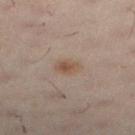Captured during whole-body skin photography for melanoma surveillance; the lesion was not biopsied. A female subject, aged 28–32. A roughly 15 mm field-of-view crop from a total-body skin photograph. The lesion's longest dimension is about 2.5 mm. The lesion is located on the left lower leg.